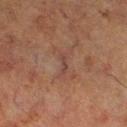No biopsy was performed on this lesion — it was imaged during a full skin examination and was not determined to be concerning.
Located on the right lower leg.
A male subject aged 63–67.
Automated tile analysis of the lesion measured a lesion area of about 3 mm², a shape eccentricity near 0.95, and a shape-asymmetry score of about 0.4 (0 = symmetric). It also reported about 5 CIELAB-L* units darker than the surrounding skin and a lesion-to-skin contrast of about 5.5 (normalized; higher = more distinct). It also reported lesion-presence confidence of about 60/100.
A region of skin cropped from a whole-body photographic capture, roughly 15 mm wide.
About 3 mm across.